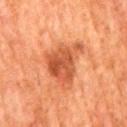Longest diameter approximately 6 mm. The lesion is located on the back. A roughly 15 mm field-of-view crop from a total-body skin photograph. Captured under cross-polarized illumination. A male patient, aged around 80.